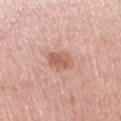Context: Captured under white-light illumination. A region of skin cropped from a whole-body photographic capture, roughly 15 mm wide. On the right upper arm. The recorded lesion diameter is about 3 mm. The subject is a female in their mid- to late 60s.